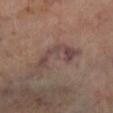{
  "biopsy_status": "not biopsied; imaged during a skin examination",
  "lighting": "cross-polarized",
  "automated_metrics": {
    "area_mm2_approx": 8.0,
    "eccentricity": 0.9,
    "shape_asymmetry": 0.7,
    "cielab_L": 41,
    "cielab_a": 16,
    "cielab_b": 18,
    "vs_skin_darker_L": 7.0,
    "vs_skin_contrast_norm": 7.0,
    "border_irregularity_0_10": 10.0,
    "color_variation_0_10": 3.0,
    "peripheral_color_asymmetry": 0.5,
    "nevus_likeness_0_100": 0,
    "lesion_detection_confidence_0_100": 75
  },
  "lesion_size": {
    "long_diameter_mm_approx": 6.0
  },
  "site": "leg",
  "image": {
    "source": "total-body photography crop",
    "field_of_view_mm": 15
  },
  "patient": {
    "sex": "male",
    "age_approx": 70
  }
}